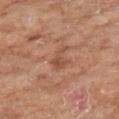Impression:
Part of a total-body skin-imaging series; this lesion was reviewed on a skin check and was not flagged for biopsy.
Context:
This image is a 15 mm lesion crop taken from a total-body photograph. About 3 mm across. A female patient aged 73 to 77. The tile uses white-light illumination. From the arm.